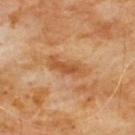Impression: No biopsy was performed on this lesion — it was imaged during a full skin examination and was not determined to be concerning. Context: This is a cross-polarized tile. The lesion-visualizer software estimated a footprint of about 8.5 mm², an eccentricity of roughly 0.95, and a symmetry-axis asymmetry near 0.3. The software also gave a mean CIELAB color near L≈52 a*≈23 b*≈39 and a lesion–skin lightness drop of about 9. And it measured border irregularity of about 4 on a 0–10 scale, a within-lesion color-variation index near 3.5/10, and peripheral color asymmetry of about 1. A male patient, aged around 60. From the chest. A close-up tile cropped from a whole-body skin photograph, about 15 mm across.